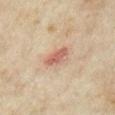{"patient": {"sex": "female", "age_approx": 35}, "image": {"source": "total-body photography crop", "field_of_view_mm": 15}, "lighting": "cross-polarized", "site": "left upper arm", "lesion_size": {"long_diameter_mm_approx": 4.0}, "automated_metrics": {"area_mm2_approx": 5.5, "eccentricity": 0.9, "shape_asymmetry": 0.25, "cielab_L": 58, "cielab_a": 22, "cielab_b": 28, "vs_skin_contrast_norm": 7.0, "border_irregularity_0_10": 3.0, "color_variation_0_10": 4.0, "peripheral_color_asymmetry": 1.5}}